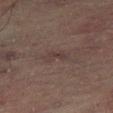Assessment: Imaged during a routine full-body skin examination; the lesion was not biopsied and no histopathology is available. Context: Cropped from a whole-body photographic skin survey; the tile spans about 15 mm. Located on the leg. This is a cross-polarized tile. A male patient, about 60 years old.